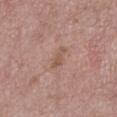Q: Was this lesion biopsied?
A: imaged on a skin check; not biopsied
Q: Where on the body is the lesion?
A: the chest
Q: Illumination type?
A: white-light illumination
Q: Patient demographics?
A: male, in their mid- to late 60s
Q: What did automated image analysis measure?
A: a lesion color around L≈54 a*≈20 b*≈27 in CIELAB
Q: What is the lesion's diameter?
A: ~3 mm (longest diameter)
Q: What is the imaging modality?
A: ~15 mm tile from a whole-body skin photo